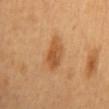Clinical impression: No biopsy was performed on this lesion — it was imaged during a full skin examination and was not determined to be concerning. Clinical summary: Cropped from a total-body skin-imaging series; the visible field is about 15 mm. The lesion is on the mid back. The patient is a female approximately 60 years of age.